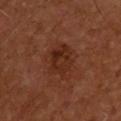The lesion was tiled from a total-body skin photograph and was not biopsied. The tile uses cross-polarized illumination. A male patient in their mid-50s. About 4 mm across. An algorithmic analysis of the crop reported a footprint of about 9.5 mm² and an eccentricity of roughly 0.65. And it measured a border-irregularity rating of about 2/10, a color-variation rating of about 4/10, and peripheral color asymmetry of about 1.5. A lesion tile, about 15 mm wide, cut from a 3D total-body photograph. Located on the back.